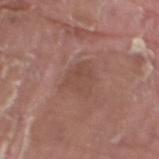This lesion was catalogued during total-body skin photography and was not selected for biopsy. Approximately 4 mm at its widest. A 15 mm close-up extracted from a 3D total-body photography capture. The lesion is located on the right thigh. An algorithmic analysis of the crop reported a mean CIELAB color near L≈47 a*≈20 b*≈25, a lesion–skin lightness drop of about 6, and a lesion-to-skin contrast of about 4.5 (normalized; higher = more distinct). The analysis additionally found an automated nevus-likeness rating near 0 out of 100 and a detector confidence of about 100 out of 100 that the crop contains a lesion. A male patient in their 40s. Imaged with white-light lighting.